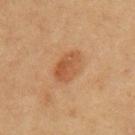The lesion was tiled from a total-body skin photograph and was not biopsied. The subject is a female about 50 years old. Located on the left upper arm. This image is a 15 mm lesion crop taken from a total-body photograph.